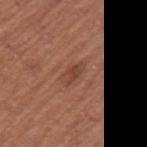biopsy status=imaged on a skin check; not biopsied
anatomic site=the left upper arm
subject=female, aged approximately 65
tile lighting=white-light
image source=total-body-photography crop, ~15 mm field of view
lesion size=≈2.5 mm